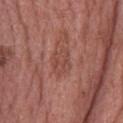workup=no biopsy performed (imaged during a skin exam) | diameter=~3 mm (longest diameter) | image=~15 mm tile from a whole-body skin photo | automated lesion analysis=an area of roughly 5 mm², an eccentricity of roughly 0.8, and two-axis asymmetry of about 0.25; about 6 CIELAB-L* units darker than the surrounding skin and a normalized lesion–skin contrast near 4.5; internal color variation of about 2 on a 0–10 scale and radial color variation of about 1; lesion-presence confidence of about 90/100 | body site=the head or neck | lighting=white-light | subject=female, aged approximately 60.